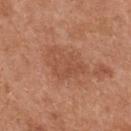| key | value |
|---|---|
| workup | no biopsy performed (imaged during a skin exam) |
| tile lighting | white-light |
| image source | ~15 mm tile from a whole-body skin photo |
| patient | female, approximately 40 years of age |
| site | the upper back |